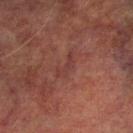follow-up: no biopsy performed (imaged during a skin exam)
anatomic site: the left lower leg
acquisition: ~15 mm crop, total-body skin-cancer survey
diameter: about 3.5 mm
automated metrics: a mean CIELAB color near L≈31 a*≈21 b*≈20, about 4 CIELAB-L* units darker than the surrounding skin, and a normalized border contrast of about 4.5; an automated nevus-likeness rating near 0 out of 100 and a detector confidence of about 80 out of 100 that the crop contains a lesion
patient: male, approximately 75 years of age